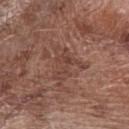This lesion was catalogued during total-body skin photography and was not selected for biopsy. Longest diameter approximately 5 mm. A 15 mm crop from a total-body photograph taken for skin-cancer surveillance. The subject is a male aged 68–72. The lesion is located on the arm.